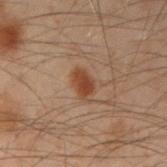Q: Was a biopsy performed?
A: total-body-photography surveillance lesion; no biopsy
Q: Automated lesion metrics?
A: a mean CIELAB color near L≈35 a*≈19 b*≈27, about 9 CIELAB-L* units darker than the surrounding skin, and a normalized border contrast of about 8.5; border irregularity of about 2.5 on a 0–10 scale, a color-variation rating of about 2.5/10, and radial color variation of about 1; a nevus-likeness score of about 95/100 and a lesion-detection confidence of about 100/100
Q: Illumination type?
A: cross-polarized illumination
Q: Where on the body is the lesion?
A: the left forearm
Q: Who is the patient?
A: male, aged around 50
Q: What is the imaging modality?
A: 15 mm crop, total-body photography
Q: How large is the lesion?
A: ≈3 mm